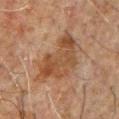Clinical impression: The lesion was tiled from a total-body skin photograph and was not biopsied. Acquisition and patient details: The lesion's longest dimension is about 7 mm. A male subject aged around 60. A 15 mm close-up tile from a total-body photography series done for melanoma screening. From the chest.